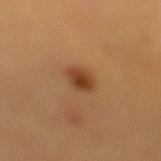Clinical impression:
Recorded during total-body skin imaging; not selected for excision or biopsy.
Context:
A female subject about 25 years old. The lesion is on the left lower leg. The lesion-visualizer software estimated a lesion-to-skin contrast of about 9 (normalized; higher = more distinct). The analysis additionally found a border-irregularity rating of about 1.5/10 and a color-variation rating of about 4/10. Imaged with cross-polarized lighting. About 2.5 mm across. A 15 mm close-up extracted from a 3D total-body photography capture.